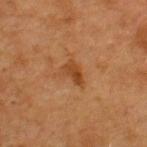Captured during whole-body skin photography for melanoma surveillance; the lesion was not biopsied. From the upper back. The recorded lesion diameter is about 3 mm. The patient is a female about 40 years old. A lesion tile, about 15 mm wide, cut from a 3D total-body photograph.